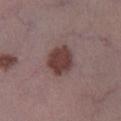Clinical impression:
The lesion was tiled from a total-body skin photograph and was not biopsied.
Background:
A male patient, aged 58 to 62. This is a white-light tile. A close-up tile cropped from a whole-body skin photograph, about 15 mm across. From the leg. The lesion's longest dimension is about 4 mm.